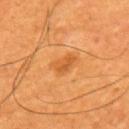Recorded during total-body skin imaging; not selected for excision or biopsy. The total-body-photography lesion software estimated a mean CIELAB color near L≈55 a*≈28 b*≈47. The software also gave border irregularity of about 3.5 on a 0–10 scale and a peripheral color-asymmetry measure near 1. Located on the back. A lesion tile, about 15 mm wide, cut from a 3D total-body photograph. Captured under cross-polarized illumination. A male patient, aged around 60.